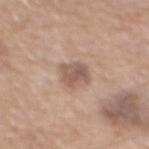Findings:
– biopsy status: no biopsy performed (imaged during a skin exam)
– anatomic site: the chest
– lesion size: ≈3.5 mm
– subject: male, aged around 70
– imaging modality: ~15 mm tile from a whole-body skin photo
– tile lighting: white-light
– automated metrics: an area of roughly 7 mm² and a shape eccentricity near 0.5; an automated nevus-likeness rating near 40 out of 100 and lesion-presence confidence of about 100/100